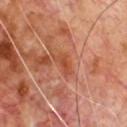Impression: No biopsy was performed on this lesion — it was imaged during a full skin examination and was not determined to be concerning. Clinical summary: A region of skin cropped from a whole-body photographic capture, roughly 15 mm wide. An algorithmic analysis of the crop reported a footprint of about 3.5 mm², an outline eccentricity of about 0.85 (0 = round, 1 = elongated), and two-axis asymmetry of about 0.35. And it measured a border-irregularity rating of about 4/10, a within-lesion color-variation index near 2/10, and radial color variation of about 0. It also reported a nevus-likeness score of about 0/100. A male patient, roughly 70 years of age. The lesion is on the chest. The recorded lesion diameter is about 3 mm. This is a cross-polarized tile.